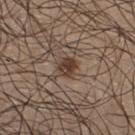biopsy status: catalogued during a skin exam; not biopsied
location: the chest
illumination: white-light illumination
image source: total-body-photography crop, ~15 mm field of view
size: ~2.5 mm (longest diameter)
patient: male, roughly 25 years of age
TBP lesion metrics: a footprint of about 5 mm² and a shape-asymmetry score of about 0.2 (0 = symmetric); a normalized lesion–skin contrast near 9.5; a nevus-likeness score of about 85/100